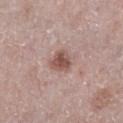This lesion was catalogued during total-body skin photography and was not selected for biopsy. Imaged with white-light lighting. The patient is a female aged 63–67. On the left lower leg. About 3 mm across. Automated image analysis of the tile measured an area of roughly 5.5 mm². And it measured a classifier nevus-likeness of about 90/100 and a detector confidence of about 100 out of 100 that the crop contains a lesion. A roughly 15 mm field-of-view crop from a total-body skin photograph.